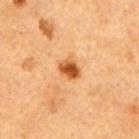Q: Was this lesion biopsied?
A: no biopsy performed (imaged during a skin exam)
Q: Where on the body is the lesion?
A: the right upper arm
Q: What is the imaging modality?
A: ~15 mm tile from a whole-body skin photo
Q: What did automated image analysis measure?
A: a mean CIELAB color near L≈49 a*≈25 b*≈40, a lesion–skin lightness drop of about 14, and a normalized border contrast of about 10.5; a border-irregularity rating of about 2/10, internal color variation of about 4.5 on a 0–10 scale, and a peripheral color-asymmetry measure near 1.5; an automated nevus-likeness rating near 100 out of 100
Q: How was the tile lit?
A: cross-polarized
Q: Patient demographics?
A: female, in their 40s
Q: Lesion size?
A: ~3 mm (longest diameter)